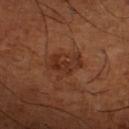This lesion was catalogued during total-body skin photography and was not selected for biopsy. A male patient in their mid-60s. Cropped from a total-body skin-imaging series; the visible field is about 15 mm. From the right lower leg.